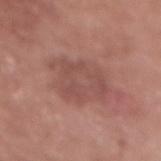This lesion was catalogued during total-body skin photography and was not selected for biopsy. On the chest. The patient is a female approximately 75 years of age. A 15 mm close-up extracted from a 3D total-body photography capture.